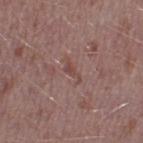The lesion was photographed on a routine skin check and not biopsied; there is no pathology result.
On the left thigh.
This image is a 15 mm lesion crop taken from a total-body photograph.
The tile uses white-light illumination.
The patient is a male aged approximately 40.